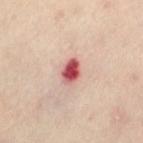No biopsy was performed on this lesion — it was imaged during a full skin examination and was not determined to be concerning.
Cropped from a total-body skin-imaging series; the visible field is about 15 mm.
A female patient, about 55 years old.
Measured at roughly 3 mm in maximum diameter.
The tile uses cross-polarized illumination.
From the leg.